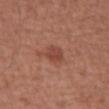Findings:
– biopsy status: no biopsy performed (imaged during a skin exam)
– site: the front of the torso
– imaging modality: ~15 mm tile from a whole-body skin photo
– lighting: white-light illumination
– subject: male, approximately 65 years of age
– automated metrics: a mean CIELAB color near L≈45 a*≈26 b*≈28, about 8 CIELAB-L* units darker than the surrounding skin, and a normalized border contrast of about 6.5